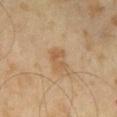| feature | finding |
|---|---|
| follow-up | catalogued during a skin exam; not biopsied |
| size | ≈2.5 mm |
| image | ~15 mm tile from a whole-body skin photo |
| patient | male, aged 63 to 67 |
| image-analysis metrics | a mean CIELAB color near L≈55 a*≈17 b*≈35, roughly 7 lightness units darker than nearby skin, and a lesion-to-skin contrast of about 5.5 (normalized; higher = more distinct); a classifier nevus-likeness of about 15/100 and a detector confidence of about 100 out of 100 that the crop contains a lesion |